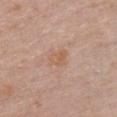Impression:
Captured during whole-body skin photography for melanoma surveillance; the lesion was not biopsied.
Background:
From the chest. This is a white-light tile. A 15 mm close-up extracted from a 3D total-body photography capture. The patient is a female aged around 50.